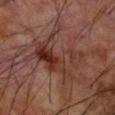{"biopsy_status": "not biopsied; imaged during a skin examination", "site": "left forearm", "patient": {"sex": "male", "age_approx": 70}, "lighting": "cross-polarized", "image": {"source": "total-body photography crop", "field_of_view_mm": 15}}